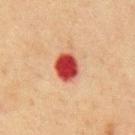Q: Is there a histopathology result?
A: no biopsy performed (imaged during a skin exam)
Q: How was this image acquired?
A: 15 mm crop, total-body photography
Q: Lesion location?
A: the abdomen
Q: Illumination type?
A: cross-polarized illumination
Q: Who is the patient?
A: male, approximately 75 years of age